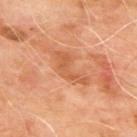biopsy status: no biopsy performed (imaged during a skin exam) | automated lesion analysis: an average lesion color of about L≈56 a*≈27 b*≈38 (CIELAB) and a normalized border contrast of about 5.5; an automated nevus-likeness rating near 0 out of 100 | site: the upper back | image source: ~15 mm crop, total-body skin-cancer survey | patient: male, aged around 65.